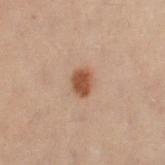<lesion>
<biopsy_status>not biopsied; imaged during a skin examination</biopsy_status>
<image>
  <source>total-body photography crop</source>
  <field_of_view_mm>15</field_of_view_mm>
</image>
<site>left thigh</site>
<automated_metrics>
  <cielab_L>40</cielab_L>
  <cielab_a>18</cielab_a>
  <cielab_b>27</cielab_b>
  <vs_skin_contrast_norm>10.0</vs_skin_contrast_norm>
  <border_irregularity_0_10>1.5</border_irregularity_0_10>
  <color_variation_0_10>2.0</color_variation_0_10>
  <peripheral_color_asymmetry>1.0</peripheral_color_asymmetry>
  <nevus_likeness_0_100>100</nevus_likeness_0_100>
  <lesion_detection_confidence_0_100>100</lesion_detection_confidence_0_100>
</automated_metrics>
<lesion_size>
  <long_diameter_mm_approx>3.0</long_diameter_mm_approx>
</lesion_size>
<patient>
  <sex>female</sex>
  <age_approx>30</age_approx>
</patient>
</lesion>